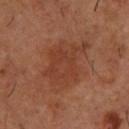Case summary:
• biopsy status · imaged on a skin check; not biopsied
• lighting · cross-polarized illumination
• image · ~15 mm tile from a whole-body skin photo
• location · the back
• subject · male, about 55 years old
• lesion diameter · ≈7 mm